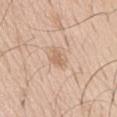Cropped from a whole-body photographic skin survey; the tile spans about 15 mm. The lesion is on the chest. A male patient, aged 68–72. This is a white-light tile.